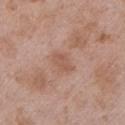Clinical impression:
No biopsy was performed on this lesion — it was imaged during a full skin examination and was not determined to be concerning.
Clinical summary:
About 2.5 mm across. The lesion is on the left upper arm. A male patient, aged 48–52. A region of skin cropped from a whole-body photographic capture, roughly 15 mm wide. An algorithmic analysis of the crop reported a mean CIELAB color near L≈55 a*≈21 b*≈29, roughly 7 lightness units darker than nearby skin, and a normalized lesion–skin contrast near 5.5. And it measured a detector confidence of about 100 out of 100 that the crop contains a lesion. This is a white-light tile.